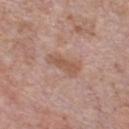<case>
<biopsy_status>not biopsied; imaged during a skin examination</biopsy_status>
<lighting>white-light</lighting>
<site>chest</site>
<patient>
  <sex>male</sex>
  <age_approx>60</age_approx>
</patient>
<image>
  <source>total-body photography crop</source>
  <field_of_view_mm>15</field_of_view_mm>
</image>
<automated_metrics>
  <cielab_L>55</cielab_L>
  <cielab_a>20</cielab_a>
  <cielab_b>29</cielab_b>
  <vs_skin_darker_L>8.0</vs_skin_darker_L>
  <vs_skin_contrast_norm>6.5</vs_skin_contrast_norm>
  <border_irregularity_0_10>3.5</border_irregularity_0_10>
  <color_variation_0_10>1.5</color_variation_0_10>
  <peripheral_color_asymmetry>0.5</peripheral_color_asymmetry>
</automated_metrics>
</case>